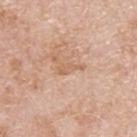This lesion was catalogued during total-body skin photography and was not selected for biopsy.
Captured under white-light illumination.
The total-body-photography lesion software estimated an area of roughly 2.5 mm², a shape eccentricity near 0.95, and a shape-asymmetry score of about 0.5 (0 = symmetric).
The patient is a male aged approximately 80.
The lesion's longest dimension is about 3 mm.
A region of skin cropped from a whole-body photographic capture, roughly 15 mm wide.
The lesion is on the upper back.